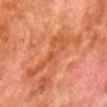Clinical impression: Imaged during a routine full-body skin examination; the lesion was not biopsied and no histopathology is available. Background: Approximately 8 mm at its widest. The lesion is located on the right lower leg. A male subject approximately 80 years of age. Imaged with cross-polarized lighting. A lesion tile, about 15 mm wide, cut from a 3D total-body photograph.